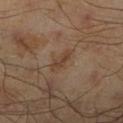Imaged during a routine full-body skin examination; the lesion was not biopsied and no histopathology is available. Located on the right lower leg. A male subject, in their mid- to late 40s. Imaged with cross-polarized lighting. A close-up tile cropped from a whole-body skin photograph, about 15 mm across. The lesion-visualizer software estimated a lesion color around L≈39 a*≈17 b*≈27 in CIELAB, a lesion–skin lightness drop of about 7, and a normalized lesion–skin contrast near 6.5. It also reported a border-irregularity rating of about 4.5/10, a within-lesion color-variation index near 0/10, and radial color variation of about 0.